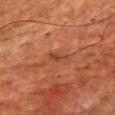Recorded during total-body skin imaging; not selected for excision or biopsy.
This is a cross-polarized tile.
Longest diameter approximately 3 mm.
From the chest.
The patient is a male aged 63–67.
An algorithmic analysis of the crop reported a lesion color around L≈35 a*≈22 b*≈29 in CIELAB and a normalized lesion–skin contrast near 6. The software also gave an automated nevus-likeness rating near 0 out of 100 and a detector confidence of about 95 out of 100 that the crop contains a lesion.
A 15 mm close-up tile from a total-body photography series done for melanoma screening.